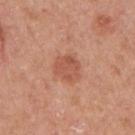{
  "biopsy_status": "not biopsied; imaged during a skin examination",
  "image": {
    "source": "total-body photography crop",
    "field_of_view_mm": 15
  },
  "site": "right upper arm",
  "patient": {
    "sex": "female",
    "age_approx": 40
  },
  "lesion_size": {
    "long_diameter_mm_approx": 3.0
  }
}